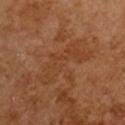Impression: Imaged during a routine full-body skin examination; the lesion was not biopsied and no histopathology is available. Acquisition and patient details: Cropped from a whole-body photographic skin survey; the tile spans about 15 mm. A male patient, in their mid-60s.